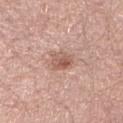Notes:
• biopsy status · no biopsy performed (imaged during a skin exam)
• patient · male, aged approximately 45
• image-analysis metrics · a lesion area of about 5 mm²
• body site · the right lower leg
• acquisition · 15 mm crop, total-body photography
• illumination · white-light illumination
• size · ≈2.5 mm